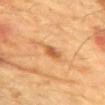Q: What did automated image analysis measure?
A: an area of roughly 3.5 mm², an eccentricity of roughly 0.8, and two-axis asymmetry of about 0.25; a mean CIELAB color near L≈51 a*≈23 b*≈38 and roughly 10 lightness units darker than nearby skin; border irregularity of about 2.5 on a 0–10 scale, a color-variation rating of about 3/10, and peripheral color asymmetry of about 1
Q: What is the anatomic site?
A: the front of the torso
Q: Illumination type?
A: cross-polarized illumination
Q: Patient demographics?
A: male, aged 83 or older
Q: How was this image acquired?
A: 15 mm crop, total-body photography